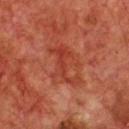  biopsy_status: not biopsied; imaged during a skin examination